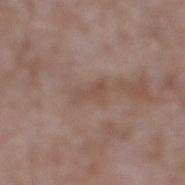Imaged during a routine full-body skin examination; the lesion was not biopsied and no histopathology is available.
A lesion tile, about 15 mm wide, cut from a 3D total-body photograph.
The subject is a male approximately 60 years of age.
About 3 mm across.
The tile uses white-light illumination.
From the right lower leg.
An algorithmic analysis of the crop reported a lesion area of about 3 mm². The analysis additionally found an average lesion color of about L≈49 a*≈18 b*≈24 (CIELAB), about 6 CIELAB-L* units darker than the surrounding skin, and a normalized lesion–skin contrast near 5.